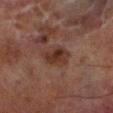{"biopsy_status": "not biopsied; imaged during a skin examination", "lighting": "cross-polarized", "lesion_size": {"long_diameter_mm_approx": 4.0}, "patient": {"sex": "male", "age_approx": 70}, "site": "left lower leg", "image": {"source": "total-body photography crop", "field_of_view_mm": 15}}